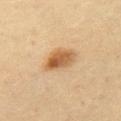- workup — catalogued during a skin exam; not biopsied
- lighting — cross-polarized
- imaging modality — total-body-photography crop, ~15 mm field of view
- anatomic site — the left upper arm
- patient — male, about 40 years old
- size — ≈4 mm